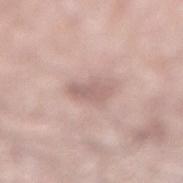notes: catalogued during a skin exam; not biopsied
illumination: white-light
image: ~15 mm crop, total-body skin-cancer survey
automated metrics: a lesion color around L≈61 a*≈18 b*≈22 in CIELAB and about 9 CIELAB-L* units darker than the surrounding skin
subject: male, aged 58 to 62
location: the right lower leg